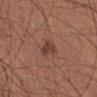No biopsy was performed on this lesion — it was imaged during a full skin examination and was not determined to be concerning. Located on the leg. Imaged with white-light lighting. A roughly 15 mm field-of-view crop from a total-body skin photograph. Automated tile analysis of the lesion measured an area of roughly 4 mm², an eccentricity of roughly 0.85, and a shape-asymmetry score of about 0.4 (0 = symmetric). The analysis additionally found a lesion color around L≈41 a*≈22 b*≈26 in CIELAB and a normalized lesion–skin contrast near 7.5. The software also gave an automated nevus-likeness rating near 80 out of 100 and a detector confidence of about 100 out of 100 that the crop contains a lesion. A male patient, approximately 55 years of age. The lesion's longest dimension is about 3 mm.